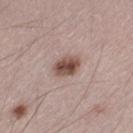The lesion was photographed on a routine skin check and not biopsied; there is no pathology result. The subject is a male approximately 45 years of age. The lesion is on the leg. A 15 mm close-up tile from a total-body photography series done for melanoma screening. Automated image analysis of the tile measured a lesion area of about 6.5 mm², an eccentricity of roughly 0.65, and a shape-asymmetry score of about 0.15 (0 = symmetric). The software also gave a classifier nevus-likeness of about 95/100.